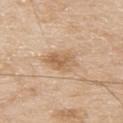• imaging modality — ~15 mm crop, total-body skin-cancer survey
• anatomic site — the upper back
• subject — male, roughly 80 years of age
• lesion size — ~4 mm (longest diameter)
• lighting — white-light
• image-analysis metrics — an area of roughly 8.5 mm², an eccentricity of roughly 0.65, and a symmetry-axis asymmetry near 0.2; a lesion color around L≈62 a*≈17 b*≈35 in CIELAB, a lesion–skin lightness drop of about 9, and a normalized lesion–skin contrast near 6.5; border irregularity of about 2.5 on a 0–10 scale, internal color variation of about 4.5 on a 0–10 scale, and a peripheral color-asymmetry measure near 1.5